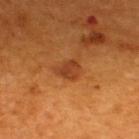<record>
  <biopsy_status>not biopsied; imaged during a skin examination</biopsy_status>
  <automated_metrics>
    <area_mm2_approx>4.5</area_mm2_approx>
    <eccentricity>0.65</eccentricity>
    <shape_asymmetry>0.4</shape_asymmetry>
    <border_irregularity_0_10>3.5</border_irregularity_0_10>
    <color_variation_0_10>2.0</color_variation_0_10>
    <peripheral_color_asymmetry>0.5</peripheral_color_asymmetry>
    <nevus_likeness_0_100>85</nevus_likeness_0_100>
  </automated_metrics>
  <patient>
    <sex>male</sex>
    <age_approx>60</age_approx>
  </patient>
  <site>upper back</site>
  <image>
    <source>total-body photography crop</source>
    <field_of_view_mm>15</field_of_view_mm>
  </image>
</record>